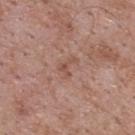notes: imaged on a skin check; not biopsied | anatomic site: the upper back | subject: male, aged 68 to 72 | image source: ~15 mm crop, total-body skin-cancer survey.